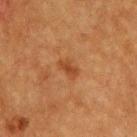Captured during whole-body skin photography for melanoma surveillance; the lesion was not biopsied. A male patient approximately 75 years of age. Cropped from a whole-body photographic skin survey; the tile spans about 15 mm. The lesion is located on the upper back.